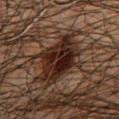<case>
<site>chest</site>
<patient>
  <sex>male</sex>
  <age_approx>60</age_approx>
</patient>
<image>
  <source>total-body photography crop</source>
  <field_of_view_mm>15</field_of_view_mm>
</image>
</case>